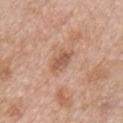biopsy status = imaged on a skin check; not biopsied | patient = female, roughly 40 years of age | image-analysis metrics = a lesion color around L≈55 a*≈22 b*≈29 in CIELAB, roughly 10 lightness units darker than nearby skin, and a normalized border contrast of about 7 | image source = ~15 mm crop, total-body skin-cancer survey | diameter = ~3 mm (longest diameter) | location = the chest.